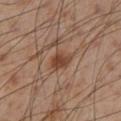Findings:
* follow-up — total-body-photography surveillance lesion; no biopsy
* body site — the leg
* image — ~15 mm tile from a whole-body skin photo
* image-analysis metrics — a nevus-likeness score of about 90/100 and lesion-presence confidence of about 100/100
* tile lighting — cross-polarized illumination
* subject — male, aged around 55